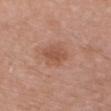Imaged during a routine full-body skin examination; the lesion was not biopsied and no histopathology is available.
The lesion is on the front of the torso.
A male subject in their 70s.
Cropped from a total-body skin-imaging series; the visible field is about 15 mm.